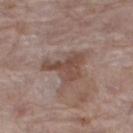Clinical impression: Part of a total-body skin-imaging series; this lesion was reviewed on a skin check and was not flagged for biopsy. Context: The tile uses white-light illumination. The lesion's longest dimension is about 5.5 mm. The lesion is located on the right thigh. A 15 mm close-up tile from a total-body photography series done for melanoma screening. A female patient, aged 83 or older.